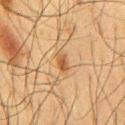The lesion was tiled from a total-body skin photograph and was not biopsied. The lesion is on the front of the torso. A male subject, roughly 60 years of age. This image is a 15 mm lesion crop taken from a total-body photograph. An algorithmic analysis of the crop reported a lesion area of about 3 mm². It also reported an average lesion color of about L≈44 a*≈18 b*≈33 (CIELAB) and a lesion–skin lightness drop of about 9. The analysis additionally found a within-lesion color-variation index near 2/10. The analysis additionally found a nevus-likeness score of about 85/100. The tile uses cross-polarized illumination. Measured at roughly 2.5 mm in maximum diameter.